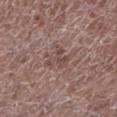<record>
  <biopsy_status>not biopsied; imaged during a skin examination</biopsy_status>
  <image>
    <source>total-body photography crop</source>
    <field_of_view_mm>15</field_of_view_mm>
  </image>
  <lesion_size>
    <long_diameter_mm_approx>4.0</long_diameter_mm_approx>
  </lesion_size>
  <automated_metrics>
    <area_mm2_approx>7.5</area_mm2_approx>
    <eccentricity>0.65</eccentricity>
    <shape_asymmetry>0.5</shape_asymmetry>
    <cielab_L>47</cielab_L>
    <cielab_a>17</cielab_a>
    <cielab_b>20</cielab_b>
    <vs_skin_darker_L>7.0</vs_skin_darker_L>
    <vs_skin_contrast_norm>5.5</vs_skin_contrast_norm>
    <nevus_likeness_0_100>0</nevus_likeness_0_100>
  </automated_metrics>
  <patient>
    <sex>male</sex>
    <age_approx>70</age_approx>
  </patient>
  <lighting>white-light</lighting>
  <site>left lower leg</site>
</record>